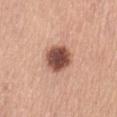follow-up: no biopsy performed (imaged during a skin exam) | subject: female, aged approximately 65 | site: the front of the torso | tile lighting: white-light illumination | automated lesion analysis: a mean CIELAB color near L≈50 a*≈23 b*≈27 and a normalized lesion–skin contrast near 12.5; border irregularity of about 1 on a 0–10 scale; a detector confidence of about 100 out of 100 that the crop contains a lesion | imaging modality: ~15 mm crop, total-body skin-cancer survey.